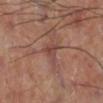This lesion was catalogued during total-body skin photography and was not selected for biopsy. The lesion is on the right lower leg. Imaged with cross-polarized lighting. This image is a 15 mm lesion crop taken from a total-body photograph. Measured at roughly 2.5 mm in maximum diameter.